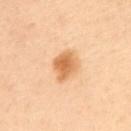The lesion was tiled from a total-body skin photograph and was not biopsied. On the mid back. Approximately 4 mm at its widest. The tile uses cross-polarized illumination. A female patient aged around 50. A roughly 15 mm field-of-view crop from a total-body skin photograph. Automated tile analysis of the lesion measured a lesion area of about 8 mm², an outline eccentricity of about 0.75 (0 = round, 1 = elongated), and a symmetry-axis asymmetry near 0.15. And it measured an average lesion color of about L≈57 a*≈20 b*≈37 (CIELAB) and roughly 11 lightness units darker than nearby skin. It also reported a color-variation rating of about 4/10 and peripheral color asymmetry of about 1. It also reported a detector confidence of about 100 out of 100 that the crop contains a lesion.